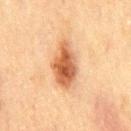workup=catalogued during a skin exam; not biopsied | subject=male, aged approximately 70 | site=the mid back | image=15 mm crop, total-body photography | illumination=cross-polarized | image-analysis metrics=an eccentricity of roughly 0.85 and a symmetry-axis asymmetry near 0.2; a peripheral color-asymmetry measure near 1.5.